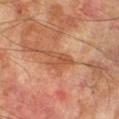Assessment: The lesion was photographed on a routine skin check and not biopsied; there is no pathology result. Background: About 4 mm across. The tile uses cross-polarized illumination. A region of skin cropped from a whole-body photographic capture, roughly 15 mm wide. From the right lower leg. Automated tile analysis of the lesion measured a lesion area of about 5.5 mm², an outline eccentricity of about 0.85 (0 = round, 1 = elongated), and a shape-asymmetry score of about 0.4 (0 = symmetric). It also reported a border-irregularity rating of about 4/10, a within-lesion color-variation index near 3.5/10, and a peripheral color-asymmetry measure near 1. A male patient aged 68–72.